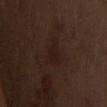The lesion was tiled from a total-body skin photograph and was not biopsied.
A male patient, about 70 years old.
A 15 mm close-up tile from a total-body photography series done for melanoma screening.
The lesion is located on the chest.